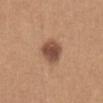Impression: Recorded during total-body skin imaging; not selected for excision or biopsy. Background: Captured under white-light illumination. A 15 mm crop from a total-body photograph taken for skin-cancer surveillance. The recorded lesion diameter is about 3 mm. Located on the abdomen. A female subject aged 33–37.